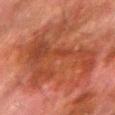Clinical impression:
The lesion was tiled from a total-body skin photograph and was not biopsied.
Acquisition and patient details:
A male subject about 80 years old. The recorded lesion diameter is about 13 mm. Captured under cross-polarized illumination. A close-up tile cropped from a whole-body skin photograph, about 15 mm across. The lesion is on the right forearm.